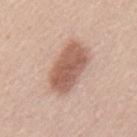notes = imaged on a skin check; not biopsied | site = the back | size = ~6 mm (longest diameter) | acquisition = 15 mm crop, total-body photography | subject = male, aged around 45.